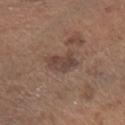biopsy status: catalogued during a skin exam; not biopsied
acquisition: 15 mm crop, total-body photography
automated metrics: a lesion area of about 6 mm², a shape eccentricity near 0.7, and a shape-asymmetry score of about 0.3 (0 = symmetric); an automated nevus-likeness rating near 5 out of 100 and a detector confidence of about 100 out of 100 that the crop contains a lesion
site: the left forearm
patient: male, aged 63 to 67
illumination: white-light illumination